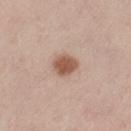Captured during whole-body skin photography for melanoma surveillance; the lesion was not biopsied. The patient is a male aged 58–62. The tile uses white-light illumination. Located on the leg. The total-body-photography lesion software estimated a mean CIELAB color near L≈55 a*≈21 b*≈29, about 14 CIELAB-L* units darker than the surrounding skin, and a lesion-to-skin contrast of about 9.5 (normalized; higher = more distinct). The software also gave a nevus-likeness score of about 100/100 and lesion-presence confidence of about 100/100. Measured at roughly 3 mm in maximum diameter. A 15 mm close-up tile from a total-body photography series done for melanoma screening.